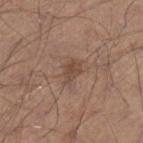The lesion was tiled from a total-body skin photograph and was not biopsied.
From the right lower leg.
A male patient, aged 28–32.
A region of skin cropped from a whole-body photographic capture, roughly 15 mm wide.
The recorded lesion diameter is about 3 mm.
Captured under white-light illumination.